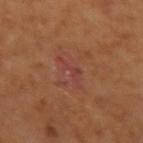| key | value |
|---|---|
| workup | imaged on a skin check; not biopsied |
| tile lighting | cross-polarized illumination |
| subject | male, in their mid-60s |
| image-analysis metrics | an outline eccentricity of about 0.95 (0 = round, 1 = elongated) and two-axis asymmetry of about 0.7; a lesion-to-skin contrast of about 5.5 (normalized; higher = more distinct); border irregularity of about 9 on a 0–10 scale, a color-variation rating of about 0/10, and radial color variation of about 0; an automated nevus-likeness rating near 0 out of 100 and a lesion-detection confidence of about 100/100 |
| image | ~15 mm tile from a whole-body skin photo |
| anatomic site | the left upper arm |
| size | about 4 mm |